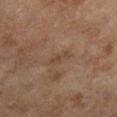Clinical impression:
No biopsy was performed on this lesion — it was imaged during a full skin examination and was not determined to be concerning.
Image and clinical context:
From the left lower leg. A female patient approximately 60 years of age. Imaged with cross-polarized lighting. A 15 mm close-up tile from a total-body photography series done for melanoma screening.